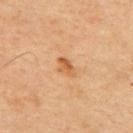Assessment: Recorded during total-body skin imaging; not selected for excision or biopsy. Image and clinical context: Captured under cross-polarized illumination. A 15 mm close-up extracted from a 3D total-body photography capture. On the upper back. The recorded lesion diameter is about 2.5 mm. The subject is a male aged 43–47.